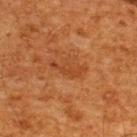Imaged during a routine full-body skin examination; the lesion was not biopsied and no histopathology is available.
The tile uses cross-polarized illumination.
Automated tile analysis of the lesion measured a mean CIELAB color near L≈37 a*≈24 b*≈34, a lesion–skin lightness drop of about 6, and a lesion-to-skin contrast of about 5 (normalized; higher = more distinct). The analysis additionally found a classifier nevus-likeness of about 0/100 and a detector confidence of about 100 out of 100 that the crop contains a lesion.
A 15 mm close-up tile from a total-body photography series done for melanoma screening.
The lesion is located on the back.
Approximately 4.5 mm at its widest.
The patient is a male aged approximately 65.